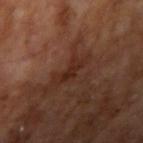Assessment:
Imaged during a routine full-body skin examination; the lesion was not biopsied and no histopathology is available.
Clinical summary:
The tile uses cross-polarized illumination. Located on the right upper arm. A region of skin cropped from a whole-body photographic capture, roughly 15 mm wide. An algorithmic analysis of the crop reported an area of roughly 3 mm², a shape eccentricity near 0.9, and a symmetry-axis asymmetry near 0.4. Approximately 3 mm at its widest. A male subject, aged around 65.